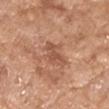workup: total-body-photography surveillance lesion; no biopsy | subject: female, about 75 years old | TBP lesion metrics: a lesion area of about 5.5 mm²; a lesion color around L≈53 a*≈23 b*≈32 in CIELAB, a lesion–skin lightness drop of about 8, and a lesion-to-skin contrast of about 6 (normalized; higher = more distinct); lesion-presence confidence of about 100/100 | lesion diameter: ≈3.5 mm | image: 15 mm crop, total-body photography | anatomic site: the right forearm | illumination: white-light.